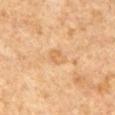follow-up = catalogued during a skin exam; not biopsied | lighting = cross-polarized | patient = male, aged around 65 | acquisition = 15 mm crop, total-body photography | body site = the chest | lesion size = ≈2.5 mm | automated metrics = a within-lesion color-variation index near 2.5/10 and a peripheral color-asymmetry measure near 1.